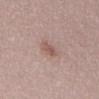* biopsy status · imaged on a skin check; not biopsied
* imaging modality · ~15 mm tile from a whole-body skin photo
* tile lighting · white-light
* site · the back
* patient · female, aged approximately 50
* lesion size · ~3 mm (longest diameter)
* TBP lesion metrics · a shape eccentricity near 0.9; an average lesion color of about L≈55 a*≈19 b*≈23 (CIELAB), a lesion–skin lightness drop of about 9, and a lesion-to-skin contrast of about 6 (normalized; higher = more distinct); lesion-presence confidence of about 100/100